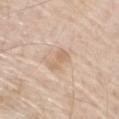Clinical impression:
The lesion was photographed on a routine skin check and not biopsied; there is no pathology result.